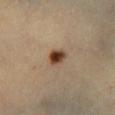Impression: Imaged during a routine full-body skin examination; the lesion was not biopsied and no histopathology is available. Acquisition and patient details: A male subject, aged 63 to 67. The tile uses cross-polarized illumination. The lesion's longest dimension is about 2.5 mm. A 15 mm crop from a total-body photograph taken for skin-cancer surveillance. Located on the left lower leg.